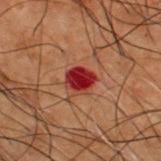The lesion was photographed on a routine skin check and not biopsied; there is no pathology result. Measured at roughly 3 mm in maximum diameter. The patient is a male approximately 50 years of age. This image is a 15 mm lesion crop taken from a total-body photograph. From the upper back. The tile uses cross-polarized illumination.